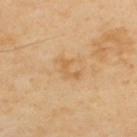This lesion was catalogued during total-body skin photography and was not selected for biopsy.
From the upper back.
A male subject roughly 55 years of age.
A region of skin cropped from a whole-body photographic capture, roughly 15 mm wide.
Captured under cross-polarized illumination.